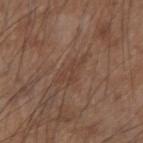The lesion was tiled from a total-body skin photograph and was not biopsied.
From the left forearm.
A male patient, approximately 55 years of age.
This image is a 15 mm lesion crop taken from a total-body photograph.
Longest diameter approximately 4.5 mm.
This is a white-light tile.
The lesion-visualizer software estimated an area of roughly 5 mm² and a shape-asymmetry score of about 0.5 (0 = symmetric). The analysis additionally found a mean CIELAB color near L≈41 a*≈17 b*≈26, roughly 5 lightness units darker than nearby skin, and a lesion-to-skin contrast of about 4.5 (normalized; higher = more distinct). It also reported a nevus-likeness score of about 0/100 and lesion-presence confidence of about 65/100.